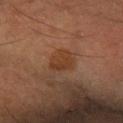Impression: The lesion was tiled from a total-body skin photograph and was not biopsied. Clinical summary: A roughly 15 mm field-of-view crop from a total-body skin photograph. This is a cross-polarized tile. From the left forearm. Longest diameter approximately 3 mm. An algorithmic analysis of the crop reported an eccentricity of roughly 0.65. And it measured about 6 CIELAB-L* units darker than the surrounding skin and a lesion-to-skin contrast of about 7 (normalized; higher = more distinct). And it measured border irregularity of about 3 on a 0–10 scale and a color-variation rating of about 2/10. And it measured a nevus-likeness score of about 20/100 and a lesion-detection confidence of about 100/100. A male subject aged around 55.